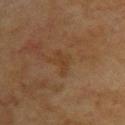Q: Is there a histopathology result?
A: total-body-photography surveillance lesion; no biopsy
Q: What did automated image analysis measure?
A: an average lesion color of about L≈32 a*≈17 b*≈29 (CIELAB) and a lesion–skin lightness drop of about 4; a border-irregularity rating of about 5/10 and peripheral color asymmetry of about 0
Q: What lighting was used for the tile?
A: cross-polarized illumination
Q: How was this image acquired?
A: 15 mm crop, total-body photography
Q: What are the patient's age and sex?
A: female, in their 80s
Q: How large is the lesion?
A: ≈2.5 mm
Q: What is the anatomic site?
A: the upper back